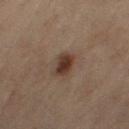A roughly 15 mm field-of-view crop from a total-body skin photograph. A female subject aged 58 to 62. From the right thigh. About 3 mm across.